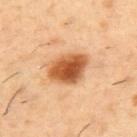{
  "site": "upper back",
  "lesion_size": {
    "long_diameter_mm_approx": 4.5
  },
  "lighting": "cross-polarized",
  "image": {
    "source": "total-body photography crop",
    "field_of_view_mm": 15
  },
  "patient": {
    "sex": "male",
    "age_approx": 55
  }
}